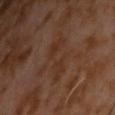The lesion was tiled from a total-body skin photograph and was not biopsied.
The lesion is located on the front of the torso.
The recorded lesion diameter is about 6 mm.
The subject is a male about 60 years old.
A close-up tile cropped from a whole-body skin photograph, about 15 mm across.
Automated tile analysis of the lesion measured a mean CIELAB color near L≈29 a*≈17 b*≈25 and a normalized lesion–skin contrast near 5.5. It also reported a nevus-likeness score of about 0/100 and a detector confidence of about 100 out of 100 that the crop contains a lesion.